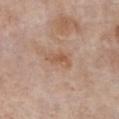| feature | finding |
|---|---|
| workup | total-body-photography surveillance lesion; no biopsy |
| image | ~15 mm crop, total-body skin-cancer survey |
| anatomic site | the chest |
| diameter | ~3.5 mm (longest diameter) |
| tile lighting | white-light |
| patient | female, in their mid-80s or older |